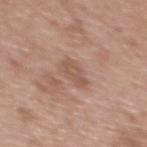Case summary:
• notes: no biopsy performed (imaged during a skin exam)
• body site: the upper back
• image source: ~15 mm crop, total-body skin-cancer survey
• patient: female, aged 38–42
• lesion diameter: ≈3.5 mm
• tile lighting: white-light
• TBP lesion metrics: a mean CIELAB color near L≈55 a*≈19 b*≈27; a border-irregularity rating of about 3/10, internal color variation of about 2.5 on a 0–10 scale, and peripheral color asymmetry of about 1; a classifier nevus-likeness of about 0/100 and a lesion-detection confidence of about 100/100